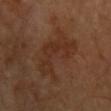Clinical impression: This lesion was catalogued during total-body skin photography and was not selected for biopsy. Background: A male subject approximately 60 years of age. From the upper back. A 15 mm close-up extracted from a 3D total-body photography capture. Automated tile analysis of the lesion measured an area of roughly 22 mm², an outline eccentricity of about 0.9 (0 = round, 1 = elongated), and a shape-asymmetry score of about 0.45 (0 = symmetric). The analysis additionally found a lesion–skin lightness drop of about 5 and a normalized border contrast of about 5.5. The analysis additionally found a border-irregularity index near 7/10, a color-variation rating of about 4/10, and radial color variation of about 1.5. And it measured an automated nevus-likeness rating near 0 out of 100 and a lesion-detection confidence of about 100/100. The recorded lesion diameter is about 8 mm.